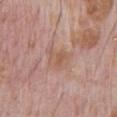Q: Is there a histopathology result?
A: catalogued during a skin exam; not biopsied
Q: Patient demographics?
A: male, aged around 70
Q: Where on the body is the lesion?
A: the front of the torso
Q: What lighting was used for the tile?
A: white-light
Q: What is the imaging modality?
A: total-body-photography crop, ~15 mm field of view